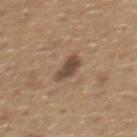Assessment:
This lesion was catalogued during total-body skin photography and was not selected for biopsy.
Clinical summary:
A 15 mm close-up extracted from a 3D total-body photography capture. From the back. About 3 mm across. Imaged with white-light lighting. A male subject, in their mid-60s. An algorithmic analysis of the crop reported a normalized lesion–skin contrast near 9.5.